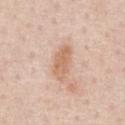Case summary:
* follow-up — no biopsy performed (imaged during a skin exam)
* acquisition — ~15 mm crop, total-body skin-cancer survey
* lighting — white-light illumination
* size — ~4.5 mm (longest diameter)
* patient — male, aged around 60
* anatomic site — the abdomen